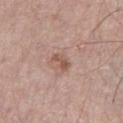No biopsy was performed on this lesion — it was imaged during a full skin examination and was not determined to be concerning.
The lesion's longest dimension is about 3 mm.
Captured under white-light illumination.
A roughly 15 mm field-of-view crop from a total-body skin photograph.
Located on the left thigh.
A male subject aged 73–77.